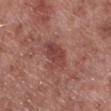biopsy_status: not biopsied; imaged during a skin examination
patient:
  sex: male
  age_approx: 55
site: right lower leg
image:
  source: total-body photography crop
  field_of_view_mm: 15
lighting: white-light